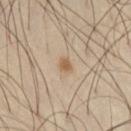Captured during whole-body skin photography for melanoma surveillance; the lesion was not biopsied. The subject is a male approximately 45 years of age. The lesion's longest dimension is about 1.5 mm. A 15 mm close-up extracted from a 3D total-body photography capture. From the right thigh.